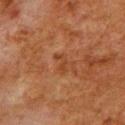follow-up = total-body-photography surveillance lesion; no biopsy | tile lighting = cross-polarized illumination | lesion size = ≈3 mm | anatomic site = the upper back | patient = male, in their 80s | acquisition = 15 mm crop, total-body photography.